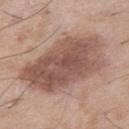No biopsy was performed on this lesion — it was imaged during a full skin examination and was not determined to be concerning.
The lesion is located on the right thigh.
A region of skin cropped from a whole-body photographic capture, roughly 15 mm wide.
The patient is a male roughly 50 years of age.
This is a white-light tile.
Approximately 10.5 mm at its widest.